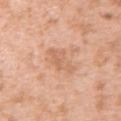Q: Was a biopsy performed?
A: catalogued during a skin exam; not biopsied
Q: Where on the body is the lesion?
A: the left upper arm
Q: How large is the lesion?
A: ≈4 mm
Q: How was the tile lit?
A: white-light
Q: What are the patient's age and sex?
A: female, roughly 40 years of age
Q: What kind of image is this?
A: 15 mm crop, total-body photography